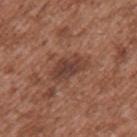Assessment:
Imaged during a routine full-body skin examination; the lesion was not biopsied and no histopathology is available.
Context:
A male patient roughly 45 years of age. A lesion tile, about 15 mm wide, cut from a 3D total-body photograph. Located on the arm. The recorded lesion diameter is about 4.5 mm.